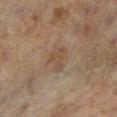This lesion was catalogued during total-body skin photography and was not selected for biopsy. A female patient aged 58–62. Located on the left lower leg. Cropped from a whole-body photographic skin survey; the tile spans about 15 mm.